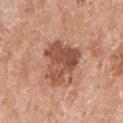notes: catalogued during a skin exam; not biopsied | site: the arm | subject: female, about 75 years old | size: ≈5 mm | illumination: white-light illumination | acquisition: total-body-photography crop, ~15 mm field of view.